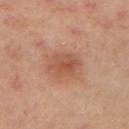Part of a total-body skin-imaging series; this lesion was reviewed on a skin check and was not flagged for biopsy.